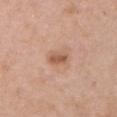follow-up = imaged on a skin check; not biopsied
tile lighting = white-light
body site = the chest
image-analysis metrics = a symmetry-axis asymmetry near 0.3; a mean CIELAB color near L≈57 a*≈22 b*≈31, roughly 10 lightness units darker than nearby skin, and a normalized lesion–skin contrast near 7; border irregularity of about 2.5 on a 0–10 scale, a color-variation rating of about 4/10, and a peripheral color-asymmetry measure near 1.5
image = ~15 mm crop, total-body skin-cancer survey
subject = female, in their 60s
lesion size = ~3 mm (longest diameter)